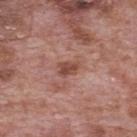Case summary:
- automated metrics — a lesion color around L≈47 a*≈24 b*≈27 in CIELAB and about 10 CIELAB-L* units darker than the surrounding skin; a border-irregularity rating of about 2.5/10 and a within-lesion color-variation index near 2/10; a classifier nevus-likeness of about 10/100 and a lesion-detection confidence of about 100/100
- imaging modality — ~15 mm crop, total-body skin-cancer survey
- diameter — ~3 mm (longest diameter)
- subject — male, aged 68–72
- body site — the mid back